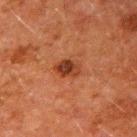* biopsy status: imaged on a skin check; not biopsied
* image-analysis metrics: lesion-presence confidence of about 100/100
* illumination: cross-polarized illumination
* patient: male, aged 58–62
* lesion size: ≈3 mm
* imaging modality: 15 mm crop, total-body photography
* body site: the arm